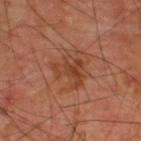Clinical impression: No biopsy was performed on this lesion — it was imaged during a full skin examination and was not determined to be concerning. Background: About 4 mm across. A region of skin cropped from a whole-body photographic capture, roughly 15 mm wide. Automated tile analysis of the lesion measured a lesion area of about 7.5 mm² and an outline eccentricity of about 0.7 (0 = round, 1 = elongated). And it measured border irregularity of about 7.5 on a 0–10 scale, a color-variation rating of about 3/10, and radial color variation of about 1. It also reported an automated nevus-likeness rating near 5 out of 100. The tile uses cross-polarized illumination. A male subject aged 58 to 62. Located on the upper back.